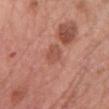workup = no biopsy performed (imaged during a skin exam); diameter = ~3 mm (longest diameter); subject = female, aged approximately 60; site = the chest; illumination = white-light illumination; acquisition = 15 mm crop, total-body photography.